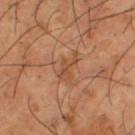Notes:
- notes: no biopsy performed (imaged during a skin exam)
- lesion size: ≈3.5 mm
- automated metrics: a lesion area of about 4 mm² and an outline eccentricity of about 0.9 (0 = round, 1 = elongated); border irregularity of about 7 on a 0–10 scale, a color-variation rating of about 0.5/10, and a peripheral color-asymmetry measure near 0; a classifier nevus-likeness of about 5/100 and a detector confidence of about 100 out of 100 that the crop contains a lesion
- illumination: cross-polarized
- imaging modality: ~15 mm tile from a whole-body skin photo
- subject: male, in their mid- to late 60s
- site: the left thigh